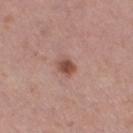lighting: white-light illumination
automated lesion analysis: a footprint of about 4 mm², an eccentricity of roughly 0.7, and two-axis asymmetry of about 0.15; an average lesion color of about L≈50 a*≈22 b*≈26 (CIELAB), about 12 CIELAB-L* units darker than the surrounding skin, and a normalized lesion–skin contrast near 8.5; a border-irregularity rating of about 1.5/10, a within-lesion color-variation index near 3/10, and radial color variation of about 1
location: the right thigh
patient: female, in their mid- to late 50s
image: ~15 mm crop, total-body skin-cancer survey